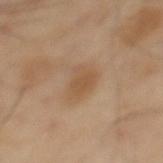{
  "patient": {
    "sex": "male",
    "age_approx": 40
  },
  "lesion_size": {
    "long_diameter_mm_approx": 3.5
  },
  "image": {
    "source": "total-body photography crop",
    "field_of_view_mm": 15
  },
  "site": "mid back",
  "automated_metrics": {
    "eccentricity": 0.7,
    "shape_asymmetry": 0.3,
    "cielab_L": 52,
    "cielab_a": 17,
    "cielab_b": 33,
    "vs_skin_contrast_norm": 6.0,
    "border_irregularity_0_10": 3.0
  },
  "lighting": "cross-polarized"
}